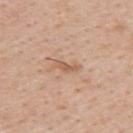From the upper back. A male subject, approximately 40 years of age. A lesion tile, about 15 mm wide, cut from a 3D total-body photograph. This is a white-light tile. Automated image analysis of the tile measured a color-variation rating of about 0/10 and peripheral color asymmetry of about 0. And it measured a nevus-likeness score of about 0/100 and a detector confidence of about 100 out of 100 that the crop contains a lesion.